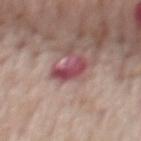| key | value |
|---|---|
| follow-up | no biopsy performed (imaged during a skin exam) |
| lesion size | ~3.5 mm (longest diameter) |
| automated lesion analysis | an area of roughly 6 mm², an eccentricity of roughly 0.8, and a symmetry-axis asymmetry near 0.45 |
| location | the mid back |
| acquisition | 15 mm crop, total-body photography |
| lighting | white-light |
| subject | male, in their mid- to late 70s |